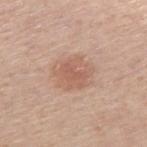biopsy status=no biopsy performed (imaged during a skin exam) | subject=male, in their 60s | image source=total-body-photography crop, ~15 mm field of view | tile lighting=white-light | anatomic site=the right upper arm.